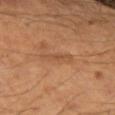Impression:
Imaged during a routine full-body skin examination; the lesion was not biopsied and no histopathology is available.
Acquisition and patient details:
Captured under cross-polarized illumination. Cropped from a total-body skin-imaging series; the visible field is about 15 mm. The patient is a male in their mid-40s. The lesion's longest dimension is about 3.5 mm. The lesion-visualizer software estimated a border-irregularity rating of about 4/10. The analysis additionally found a classifier nevus-likeness of about 0/100. On the left forearm.